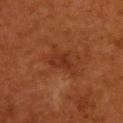The lesion is located on the upper back.
Automated tile analysis of the lesion measured a nevus-likeness score of about 0/100 and lesion-presence confidence of about 100/100.
A region of skin cropped from a whole-body photographic capture, roughly 15 mm wide.
The tile uses cross-polarized illumination.
A male patient, approximately 60 years of age.
The recorded lesion diameter is about 3 mm.